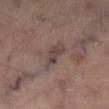Part of a total-body skin-imaging series; this lesion was reviewed on a skin check and was not flagged for biopsy.
Measured at roughly 3.5 mm in maximum diameter.
Imaged with cross-polarized lighting.
The subject is a male approximately 70 years of age.
A roughly 15 mm field-of-view crop from a total-body skin photograph.
Located on the left lower leg.